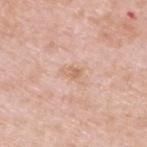{"biopsy_status": "not biopsied; imaged during a skin examination", "lesion_size": {"long_diameter_mm_approx": 3.0}, "site": "upper back", "image": {"source": "total-body photography crop", "field_of_view_mm": 15}, "patient": {"sex": "male", "age_approx": 50}, "lighting": "white-light"}